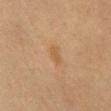notes: no biopsy performed (imaged during a skin exam)
acquisition: total-body-photography crop, ~15 mm field of view
lesion diameter: ≈3 mm
site: the chest
patient: female, roughly 80 years of age
automated metrics: a lesion area of about 3.5 mm², a shape eccentricity near 0.85, and a symmetry-axis asymmetry near 0.25; border irregularity of about 2.5 on a 0–10 scale, internal color variation of about 1 on a 0–10 scale, and peripheral color asymmetry of about 0; an automated nevus-likeness rating near 30 out of 100
tile lighting: cross-polarized